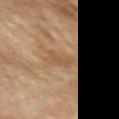The lesion was photographed on a routine skin check and not biopsied; there is no pathology result.
The lesion is located on the arm.
A female subject, aged 68 to 72.
Longest diameter approximately 3.5 mm.
Automated image analysis of the tile measured a lesion area of about 5 mm², an outline eccentricity of about 0.8 (0 = round, 1 = elongated), and a shape-asymmetry score of about 0.3 (0 = symmetric). And it measured an average lesion color of about L≈55 a*≈18 b*≈34 (CIELAB), roughly 7 lightness units darker than nearby skin, and a normalized border contrast of about 5. The analysis additionally found a border-irregularity rating of about 3/10 and internal color variation of about 1.5 on a 0–10 scale.
Cropped from a whole-body photographic skin survey; the tile spans about 15 mm.
Captured under cross-polarized illumination.